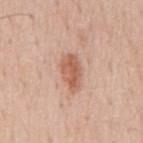<case>
<biopsy_status>not biopsied; imaged during a skin examination</biopsy_status>
<patient>
  <sex>male</sex>
  <age_approx>60</age_approx>
</patient>
<image>
  <source>total-body photography crop</source>
  <field_of_view_mm>15</field_of_view_mm>
</image>
<site>back</site>
<lighting>white-light</lighting>
</case>